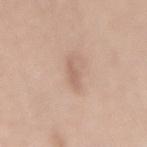Captured during whole-body skin photography for melanoma surveillance; the lesion was not biopsied. A 15 mm crop from a total-body photograph taken for skin-cancer surveillance. An algorithmic analysis of the crop reported a footprint of about 2.5 mm² and an eccentricity of roughly 0.95. And it measured a nevus-likeness score of about 0/100 and a detector confidence of about 100 out of 100 that the crop contains a lesion. The lesion's longest dimension is about 3 mm. The lesion is on the mid back. Captured under white-light illumination. A female subject, aged 58 to 62.